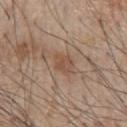Notes:
– workup: total-body-photography surveillance lesion; no biopsy
– lighting: white-light illumination
– body site: the chest
– diameter: about 2.5 mm
– subject: male, aged 58–62
– image: 15 mm crop, total-body photography